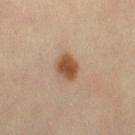A lesion tile, about 15 mm wide, cut from a 3D total-body photograph. The lesion-visualizer software estimated a border-irregularity rating of about 2/10, internal color variation of about 2.5 on a 0–10 scale, and a peripheral color-asymmetry measure near 0.5. On the leg. The subject is a female in their mid- to late 40s. Measured at roughly 3 mm in maximum diameter.